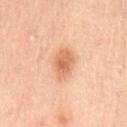From the lower back. A 15 mm close-up tile from a total-body photography series done for melanoma screening. A male subject, approximately 65 years of age. Automated image analysis of the tile measured an area of roughly 9 mm² and two-axis asymmetry of about 0.2. And it measured border irregularity of about 1.5 on a 0–10 scale. The software also gave an automated nevus-likeness rating near 90 out of 100 and a lesion-detection confidence of about 100/100. Imaged with cross-polarized lighting. The recorded lesion diameter is about 4 mm.